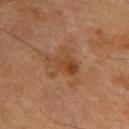follow-up — no biopsy performed (imaged during a skin exam) | imaging modality — 15 mm crop, total-body photography | subject — male, about 70 years old | tile lighting — cross-polarized | lesion diameter — ≈4 mm | automated lesion analysis — a footprint of about 6.5 mm² and an outline eccentricity of about 0.8 (0 = round, 1 = elongated); a mean CIELAB color near L≈43 a*≈22 b*≈34 and a lesion-to-skin contrast of about 6.5 (normalized; higher = more distinct); a border-irregularity rating of about 5.5/10, a within-lesion color-variation index near 3/10, and a peripheral color-asymmetry measure near 1 | body site — the upper back.